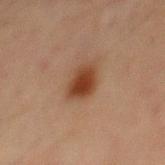The lesion was tiled from a total-body skin photograph and was not biopsied. The subject is a male aged approximately 70. On the abdomen. A 15 mm close-up extracted from a 3D total-body photography capture.